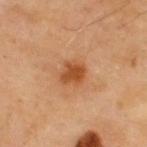{
  "biopsy_status": "not biopsied; imaged during a skin examination",
  "patient": {
    "sex": "male",
    "age_approx": 40
  },
  "site": "upper back",
  "lighting": "cross-polarized",
  "lesion_size": {
    "long_diameter_mm_approx": 3.0
  },
  "image": {
    "source": "total-body photography crop",
    "field_of_view_mm": 15
  }
}